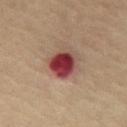Captured during whole-body skin photography for melanoma surveillance; the lesion was not biopsied. The patient is a male approximately 70 years of age. A region of skin cropped from a whole-body photographic capture, roughly 15 mm wide. From the chest.